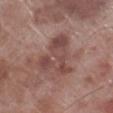Captured during whole-body skin photography for melanoma surveillance; the lesion was not biopsied. From the right lower leg. A male subject, aged 68–72. Cropped from a total-body skin-imaging series; the visible field is about 15 mm.